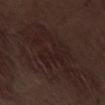biopsy status: catalogued during a skin exam; not biopsied
site: the leg
subject: male, in their 70s
imaging modality: 15 mm crop, total-body photography
tile lighting: white-light illumination
lesion size: about 11 mm